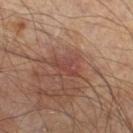Imaged during a routine full-body skin examination; the lesion was not biopsied and no histopathology is available.